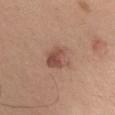Recorded during total-body skin imaging; not selected for excision or biopsy. Cropped from a whole-body photographic skin survey; the tile spans about 15 mm. The lesion is located on the upper back. A male patient, approximately 55 years of age.